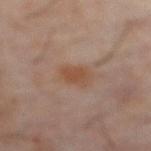Q: How was this image acquired?
A: total-body-photography crop, ~15 mm field of view
Q: Where on the body is the lesion?
A: the abdomen
Q: What are the patient's age and sex?
A: male, roughly 60 years of age